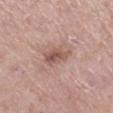Acquisition and patient details: A 15 mm close-up extracted from a 3D total-body photography capture. This is a white-light tile. The lesion-visualizer software estimated a mean CIELAB color near L≈54 a*≈20 b*≈24, a lesion–skin lightness drop of about 11, and a lesion-to-skin contrast of about 7.5 (normalized; higher = more distinct). The analysis additionally found a border-irregularity index near 3/10, a within-lesion color-variation index near 5/10, and a peripheral color-asymmetry measure near 2. The lesion is on the right lower leg. Measured at roughly 3.5 mm in maximum diameter. The patient is a female in their mid- to late 50s.